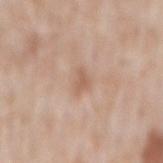| field | value |
|---|---|
| follow-up | total-body-photography surveillance lesion; no biopsy |
| subject | male, aged 48 to 52 |
| site | the mid back |
| image | total-body-photography crop, ~15 mm field of view |